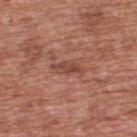| field | value |
|---|---|
| biopsy status | total-body-photography surveillance lesion; no biopsy |
| automated lesion analysis | a border-irregularity index near 3.5/10 and a within-lesion color-variation index near 2.5/10 |
| image | ~15 mm tile from a whole-body skin photo |
| body site | the upper back |
| illumination | white-light illumination |
| size | ~4 mm (longest diameter) |
| patient | male, roughly 70 years of age |